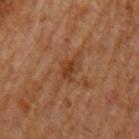  biopsy_status: not biopsied; imaged during a skin examination
  patient:
    sex: male
    age_approx: 65
  image:
    source: total-body photography crop
    field_of_view_mm: 15
  site: arm
  lighting: cross-polarized
  lesion_size:
    long_diameter_mm_approx: 3.0